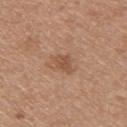| feature | finding |
|---|---|
| biopsy status | imaged on a skin check; not biopsied |
| location | the upper back |
| automated lesion analysis | a lesion color around L≈51 a*≈20 b*≈32 in CIELAB, roughly 9 lightness units darker than nearby skin, and a normalized lesion–skin contrast near 6.5; an automated nevus-likeness rating near 15 out of 100 and lesion-presence confidence of about 100/100 |
| size | ≈2.5 mm |
| acquisition | ~15 mm crop, total-body skin-cancer survey |
| subject | female, roughly 45 years of age |